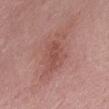  patient:
    sex: female
    age_approx: 50
  image:
    source: total-body photography crop
    field_of_view_mm: 15
  site: abdomen
  lighting: white-light
  lesion_size:
    long_diameter_mm_approx: 3.0